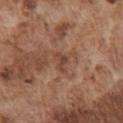Acquisition and patient details:
A male subject aged around 75. On the chest. This image is a 15 mm lesion crop taken from a total-body photograph. Imaged with white-light lighting. Approximately 3 mm at its widest.